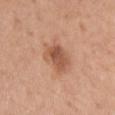Q: Was this lesion biopsied?
A: total-body-photography surveillance lesion; no biopsy
Q: Patient demographics?
A: male, roughly 35 years of age
Q: What is the imaging modality?
A: 15 mm crop, total-body photography
Q: Lesion location?
A: the chest
Q: Lesion size?
A: about 3.5 mm
Q: What lighting was used for the tile?
A: white-light